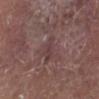subject = male, aged 78–82 | body site = the left lower leg | automated metrics = roughly 6 lightness units darker than nearby skin and a normalized lesion–skin contrast near 5.5; internal color variation of about 2.5 on a 0–10 scale and radial color variation of about 1 | acquisition = 15 mm crop, total-body photography.